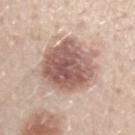Impression: No biopsy was performed on this lesion — it was imaged during a full skin examination and was not determined to be concerning. Context: A male patient aged 28 to 32. This is a white-light tile. The lesion's longest dimension is about 7 mm. Automated image analysis of the tile measured a mean CIELAB color near L≈57 a*≈20 b*≈24 and a normalized lesion–skin contrast near 10. The software also gave a border-irregularity rating of about 2/10 and a within-lesion color-variation index near 6/10. From the left forearm. A region of skin cropped from a whole-body photographic capture, roughly 15 mm wide.